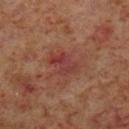| feature | finding |
|---|---|
| biopsy status | catalogued during a skin exam; not biopsied |
| automated metrics | an area of roughly 6 mm², an outline eccentricity of about 0.8 (0 = round, 1 = elongated), and a shape-asymmetry score of about 0.3 (0 = symmetric); border irregularity of about 3.5 on a 0–10 scale and internal color variation of about 7.5 on a 0–10 scale; a classifier nevus-likeness of about 0/100 and a detector confidence of about 100 out of 100 that the crop contains a lesion |
| size | ≈3.5 mm |
| body site | the leg |
| patient | male, aged 58 to 62 |
| imaging modality | total-body-photography crop, ~15 mm field of view |
| illumination | cross-polarized illumination |